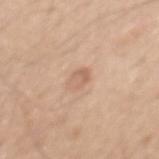Assessment:
Imaged during a routine full-body skin examination; the lesion was not biopsied and no histopathology is available.
Clinical summary:
Captured under white-light illumination. Automated image analysis of the tile measured a footprint of about 4 mm² and a symmetry-axis asymmetry near 0.15. The analysis additionally found a border-irregularity rating of about 1.5/10, a within-lesion color-variation index near 3.5/10, and peripheral color asymmetry of about 1.5. From the mid back. The subject is a male in their mid-40s. A 15 mm close-up tile from a total-body photography series done for melanoma screening.